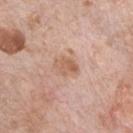biopsy status: total-body-photography surveillance lesion; no biopsy
size: ~3 mm (longest diameter)
acquisition: total-body-photography crop, ~15 mm field of view
tile lighting: white-light
subject: male, about 70 years old
body site: the front of the torso
automated lesion analysis: an area of roughly 5 mm² and an outline eccentricity of about 0.7 (0 = round, 1 = elongated); a lesion color around L≈60 a*≈20 b*≈31 in CIELAB and a normalized lesion–skin contrast near 6.5; a border-irregularity index near 2/10 and radial color variation of about 1.5; an automated nevus-likeness rating near 5 out of 100 and a detector confidence of about 100 out of 100 that the crop contains a lesion